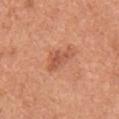Impression:
Captured during whole-body skin photography for melanoma surveillance; the lesion was not biopsied.
Image and clinical context:
Cropped from a total-body skin-imaging series; the visible field is about 15 mm. The lesion's longest dimension is about 3.5 mm. Imaged with white-light lighting. The patient is a male aged around 65. The lesion is located on the chest.